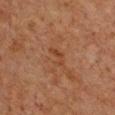Assessment: Captured during whole-body skin photography for melanoma surveillance; the lesion was not biopsied. Context: The tile uses cross-polarized illumination. An algorithmic analysis of the crop reported a normalized border contrast of about 5. And it measured internal color variation of about 0 on a 0–10 scale and radial color variation of about 0. This image is a 15 mm lesion crop taken from a total-body photograph. Measured at roughly 3 mm in maximum diameter. A female subject, aged approximately 50. From the chest.